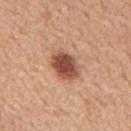patient — male, in their mid-60s
image source — 15 mm crop, total-body photography
anatomic site — the mid back
automated metrics — an area of roughly 9.5 mm², a shape eccentricity near 0.65, and a shape-asymmetry score of about 0.15 (0 = symmetric); a lesion color around L≈51 a*≈25 b*≈30 in CIELAB, roughly 17 lightness units darker than nearby skin, and a normalized border contrast of about 11; a border-irregularity index near 1.5/10 and peripheral color asymmetry of about 1
lesion diameter — ~4 mm (longest diameter)
lighting — white-light illumination